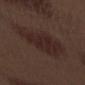Impression: The lesion was photographed on a routine skin check and not biopsied; there is no pathology result. Image and clinical context: A 15 mm close-up extracted from a 3D total-body photography capture. The lesion is on the left forearm. The subject is a male approximately 70 years of age.